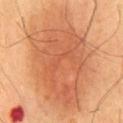Part of a total-body skin-imaging series; this lesion was reviewed on a skin check and was not flagged for biopsy.
The lesion is on the chest.
A male subject, roughly 60 years of age.
This is a cross-polarized tile.
Approximately 14 mm at its widest.
A 15 mm close-up extracted from a 3D total-body photography capture.